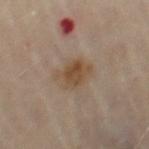Imaged during a routine full-body skin examination; the lesion was not biopsied and no histopathology is available. A female subject about 60 years old. From the right thigh. This image is a 15 mm lesion crop taken from a total-body photograph.